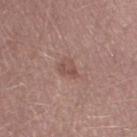Imaged with white-light lighting. The recorded lesion diameter is about 3 mm. Cropped from a total-body skin-imaging series; the visible field is about 15 mm. The patient is a male aged approximately 30.